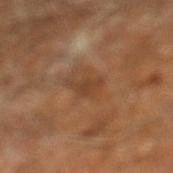Notes:
- acquisition — total-body-photography crop, ~15 mm field of view
- TBP lesion metrics — a footprint of about 3.5 mm², a shape eccentricity near 0.85, and a shape-asymmetry score of about 0.55 (0 = symmetric); a mean CIELAB color near L≈39 a*≈19 b*≈31, roughly 7 lightness units darker than nearby skin, and a normalized border contrast of about 6; internal color variation of about 1 on a 0–10 scale and radial color variation of about 0.5
- patient — male, aged approximately 60
- location — the leg
- diameter — about 3 mm